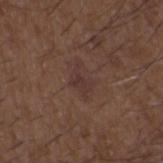Notes:
- biopsy status · catalogued during a skin exam; not biopsied